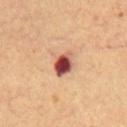automated_metrics:
  eccentricity: 0.7
  shape_asymmetry: 0.15
  nevus_likeness_0_100: 0
  lesion_detection_confidence_0_100: 100
image:
  source: total-body photography crop
  field_of_view_mm: 15
patient:
  sex: male
  age_approx: 65
lesion_size:
  long_diameter_mm_approx: 3.0
site: mid back